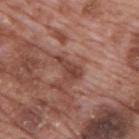Recorded during total-body skin imaging; not selected for excision or biopsy. A male patient, in their 70s. A 15 mm close-up extracted from a 3D total-body photography capture. This is a white-light tile. Longest diameter approximately 3.5 mm. The lesion is located on the back.